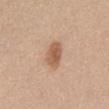Recorded during total-body skin imaging; not selected for excision or biopsy.
This image is a 15 mm lesion crop taken from a total-body photograph.
A female patient, aged 58–62.
Captured under white-light illumination.
From the abdomen.
The total-body-photography lesion software estimated a lesion color around L≈58 a*≈20 b*≈33 in CIELAB, a lesion–skin lightness drop of about 11, and a normalized border contrast of about 8. The software also gave a border-irregularity rating of about 1.5/10, a color-variation rating of about 2.5/10, and peripheral color asymmetry of about 0.5.
About 3.5 mm across.